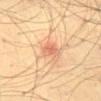• biopsy status: imaged on a skin check; not biopsied